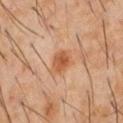biopsy status=total-body-photography surveillance lesion; no biopsy
image source=~15 mm tile from a whole-body skin photo
diameter=about 3 mm
body site=the front of the torso
image-analysis metrics=a lesion color around L≈51 a*≈24 b*≈36 in CIELAB, a lesion–skin lightness drop of about 10, and a normalized border contrast of about 8.5; border irregularity of about 2 on a 0–10 scale and radial color variation of about 1
tile lighting=cross-polarized illumination
subject=male, in their 60s